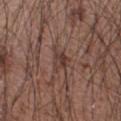{"biopsy_status": "not biopsied; imaged during a skin examination", "patient": {"sex": "male", "age_approx": 65}, "automated_metrics": {"area_mm2_approx": 5.0, "eccentricity": 0.45, "shape_asymmetry": 0.4, "cielab_L": 39, "cielab_a": 18, "cielab_b": 22, "vs_skin_darker_L": 8.0, "vs_skin_contrast_norm": 7.0, "border_irregularity_0_10": 4.0, "color_variation_0_10": 4.5, "peripheral_color_asymmetry": 2.0}, "site": "right upper arm", "lesion_size": {"long_diameter_mm_approx": 3.0}, "image": {"source": "total-body photography crop", "field_of_view_mm": 15}}